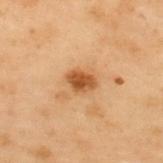workup = imaged on a skin check; not biopsied
image = ~15 mm crop, total-body skin-cancer survey
tile lighting = cross-polarized
diameter = about 3 mm
patient = male, roughly 55 years of age
site = the upper back
image-analysis metrics = a lesion area of about 5 mm², an eccentricity of roughly 0.7, and two-axis asymmetry of about 0.25; a normalized lesion–skin contrast near 9.5; a border-irregularity index near 2/10, internal color variation of about 2.5 on a 0–10 scale, and peripheral color asymmetry of about 1